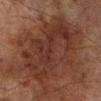Recorded during total-body skin imaging; not selected for excision or biopsy. A male subject, roughly 70 years of age. Located on the right lower leg. A close-up tile cropped from a whole-body skin photograph, about 15 mm across.